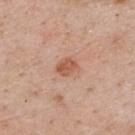{
  "biopsy_status": "not biopsied; imaged during a skin examination",
  "image": {
    "source": "total-body photography crop",
    "field_of_view_mm": 15
  },
  "patient": {
    "sex": "male",
    "age_approx": 60
  },
  "automated_metrics": {
    "area_mm2_approx": 4.5,
    "eccentricity": 0.65,
    "cielab_L": 56,
    "cielab_a": 24,
    "cielab_b": 32,
    "vs_skin_contrast_norm": 7.5
  },
  "site": "upper back",
  "lighting": "white-light"
}